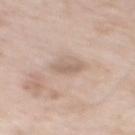Assessment: No biopsy was performed on this lesion — it was imaged during a full skin examination and was not determined to be concerning. Background: The tile uses white-light illumination. Located on the mid back. A lesion tile, about 15 mm wide, cut from a 3D total-body photograph. Approximately 3 mm at its widest. A male subject about 65 years old.